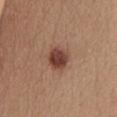Clinical impression: Captured during whole-body skin photography for melanoma surveillance; the lesion was not biopsied. Background: A region of skin cropped from a whole-body photographic capture, roughly 15 mm wide. Imaged with white-light lighting. A female patient, aged 43 to 47. From the front of the torso.